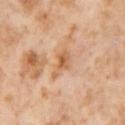The lesion was tiled from a total-body skin photograph and was not biopsied. The lesion is located on the right thigh. Imaged with cross-polarized lighting. About 4 mm across. A 15 mm close-up extracted from a 3D total-body photography capture. A female patient, aged around 55.